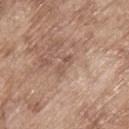Assessment:
The lesion was tiled from a total-body skin photograph and was not biopsied.
Context:
Cropped from a whole-body photographic skin survey; the tile spans about 15 mm. The total-body-photography lesion software estimated an eccentricity of roughly 0.9 and a shape-asymmetry score of about 0.25 (0 = symmetric). A male patient, aged around 65. This is a white-light tile. From the upper back. The lesion's longest dimension is about 2.5 mm.